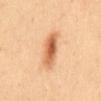Impression:
Recorded during total-body skin imaging; not selected for excision or biopsy.
Acquisition and patient details:
A female subject in their mid- to late 30s. Imaged with cross-polarized lighting. On the abdomen. The lesion-visualizer software estimated a lesion area of about 11 mm² and a shape eccentricity near 0.9. The analysis additionally found a lesion color around L≈64 a*≈24 b*≈39 in CIELAB, a lesion–skin lightness drop of about 14, and a normalized border contrast of about 8.5. The software also gave a border-irregularity index near 2.5/10, a within-lesion color-variation index near 7.5/10, and a peripheral color-asymmetry measure near 2.5. The analysis additionally found a nevus-likeness score of about 100/100 and lesion-presence confidence of about 100/100. Cropped from a whole-body photographic skin survey; the tile spans about 15 mm.